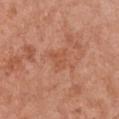Q: Is there a histopathology result?
A: total-body-photography surveillance lesion; no biopsy
Q: Where on the body is the lesion?
A: the chest
Q: What is the lesion's diameter?
A: ~2.5 mm (longest diameter)
Q: How was the tile lit?
A: white-light
Q: What is the imaging modality?
A: 15 mm crop, total-body photography
Q: Patient demographics?
A: female, about 65 years old